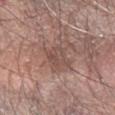Clinical impression: This lesion was catalogued during total-body skin photography and was not selected for biopsy. Clinical summary: A male subject approximately 75 years of age. This image is a 15 mm lesion crop taken from a total-body photograph. The tile uses white-light illumination. An algorithmic analysis of the crop reported a shape eccentricity near 0.5. The analysis additionally found border irregularity of about 4 on a 0–10 scale. The software also gave a classifier nevus-likeness of about 0/100. Longest diameter approximately 3 mm. On the right forearm.